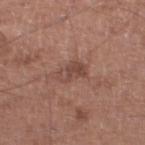No biopsy was performed on this lesion — it was imaged during a full skin examination and was not determined to be concerning. On the left lower leg. A male patient approximately 65 years of age. Longest diameter approximately 3.5 mm. The tile uses white-light illumination. Automated image analysis of the tile measured an average lesion color of about L≈45 a*≈21 b*≈24 (CIELAB) and a lesion–skin lightness drop of about 8. The software also gave a border-irregularity rating of about 3.5/10, internal color variation of about 4 on a 0–10 scale, and radial color variation of about 1.5. A 15 mm crop from a total-body photograph taken for skin-cancer surveillance.